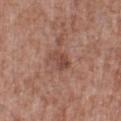Clinical impression: Recorded during total-body skin imaging; not selected for excision or biopsy. Acquisition and patient details: From the left lower leg. Measured at roughly 2.5 mm in maximum diameter. A male subject in their mid-50s. Captured under white-light illumination. This image is a 15 mm lesion crop taken from a total-body photograph.